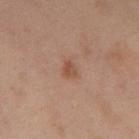- follow-up · no biopsy performed (imaged during a skin exam)
- subject · female, about 50 years old
- size · ≈2 mm
- body site · the left thigh
- acquisition · ~15 mm crop, total-body skin-cancer survey
- lighting · cross-polarized
- image-analysis metrics · an area of roughly 3 mm² and an eccentricity of roughly 0.7; a lesion color around L≈50 a*≈21 b*≈30 in CIELAB and roughly 8 lightness units darker than nearby skin; a border-irregularity index near 2.5/10 and a within-lesion color-variation index near 2/10